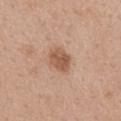Imaged during a routine full-body skin examination; the lesion was not biopsied and no histopathology is available. This image is a 15 mm lesion crop taken from a total-body photograph. The lesion is located on the mid back. About 3 mm across. The tile uses white-light illumination. A female patient, about 40 years old. The lesion-visualizer software estimated a mean CIELAB color near L≈55 a*≈21 b*≈31, about 11 CIELAB-L* units darker than the surrounding skin, and a lesion-to-skin contrast of about 8 (normalized; higher = more distinct). The analysis additionally found a border-irregularity index near 1.5/10, a color-variation rating of about 3/10, and a peripheral color-asymmetry measure near 1.